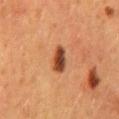Clinical impression:
Part of a total-body skin-imaging series; this lesion was reviewed on a skin check and was not flagged for biopsy.
Image and clinical context:
On the mid back. This image is a 15 mm lesion crop taken from a total-body photograph. A female subject about 50 years old. The recorded lesion diameter is about 3.5 mm. Captured under cross-polarized illumination.